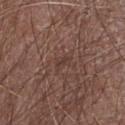Q: Is there a histopathology result?
A: catalogued during a skin exam; not biopsied
Q: How large is the lesion?
A: ≈2.5 mm
Q: What are the patient's age and sex?
A: male, about 60 years old
Q: What kind of image is this?
A: total-body-photography crop, ~15 mm field of view
Q: What is the anatomic site?
A: the arm